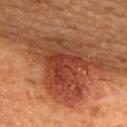<case>
<biopsy_status>not biopsied; imaged during a skin examination</biopsy_status>
<lesion_size>
  <long_diameter_mm_approx>6.0</long_diameter_mm_approx>
</lesion_size>
<image>
  <source>total-body photography crop</source>
  <field_of_view_mm>15</field_of_view_mm>
</image>
<patient>
  <sex>female</sex>
  <age_approx>55</age_approx>
</patient>
<site>upper back</site>
</case>